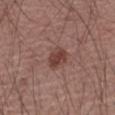notes = total-body-photography surveillance lesion; no biopsy | body site = the arm | image source = ~15 mm tile from a whole-body skin photo | patient = male, roughly 50 years of age.